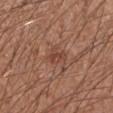notes — total-body-photography surveillance lesion; no biopsy | automated lesion analysis — a lesion color around L≈44 a*≈23 b*≈28 in CIELAB and about 8 CIELAB-L* units darker than the surrounding skin; an automated nevus-likeness rating near 5 out of 100 and a lesion-detection confidence of about 100/100 | location — the left forearm | size — about 3 mm | illumination — white-light | subject — male, roughly 30 years of age | image — total-body-photography crop, ~15 mm field of view.